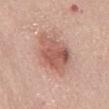{"biopsy_status": "not biopsied; imaged during a skin examination", "image": {"source": "total-body photography crop", "field_of_view_mm": 15}, "automated_metrics": {"nevus_likeness_0_100": 85, "lesion_detection_confidence_0_100": 100}, "site": "mid back", "patient": {"sex": "female", "age_approx": 65}, "lighting": "white-light", "lesion_size": {"long_diameter_mm_approx": 7.0}}